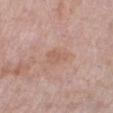| key | value |
|---|---|
| workup | total-body-photography surveillance lesion; no biopsy |
| tile lighting | white-light |
| imaging modality | total-body-photography crop, ~15 mm field of view |
| location | the right lower leg |
| automated lesion analysis | an eccentricity of roughly 0.85 and a symmetry-axis asymmetry near 0.3; a lesion color around L≈59 a*≈20 b*≈28 in CIELAB and a normalized lesion–skin contrast near 5; internal color variation of about 1.5 on a 0–10 scale and peripheral color asymmetry of about 0.5 |
| subject | female, approximately 60 years of age |